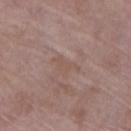biopsy status — total-body-photography surveillance lesion; no biopsy | size — ~4 mm (longest diameter) | tile lighting — white-light | acquisition — total-body-photography crop, ~15 mm field of view | patient — female, in their 70s | site — the right lower leg | automated metrics — a footprint of about 4.5 mm² and a symmetry-axis asymmetry near 0.65; a lesion color around L≈52 a*≈17 b*≈24 in CIELAB, about 5 CIELAB-L* units darker than the surrounding skin, and a lesion-to-skin contrast of about 4.5 (normalized; higher = more distinct); a classifier nevus-likeness of about 0/100 and a lesion-detection confidence of about 55/100.